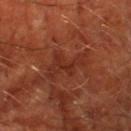  automated_metrics:
    border_irregularity_0_10: 7.5
    color_variation_0_10: 3.0
    peripheral_color_asymmetry: 1.0
    nevus_likeness_0_100: 0
    lesion_detection_confidence_0_100: 95
  patient:
    sex: male
    age_approx: 65
  site: left lower leg
  image:
    source: total-body photography crop
    field_of_view_mm: 15
  lighting: cross-polarized
  lesion_size:
    long_diameter_mm_approx: 5.0